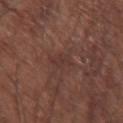Impression:
Captured during whole-body skin photography for melanoma surveillance; the lesion was not biopsied.
Acquisition and patient details:
The tile uses white-light illumination. A 15 mm crop from a total-body photograph taken for skin-cancer surveillance. A male subject, aged 63–67. Longest diameter approximately 3 mm. The lesion is on the right upper arm.